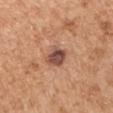Clinical impression:
This lesion was catalogued during total-body skin photography and was not selected for biopsy.
Acquisition and patient details:
Located on the left upper arm. A male subject about 65 years old. A 15 mm close-up extracted from a 3D total-body photography capture. The total-body-photography lesion software estimated a lesion area of about 6.5 mm², a shape eccentricity near 0.5, and a symmetry-axis asymmetry near 0.15. And it measured a lesion color around L≈50 a*≈22 b*≈28 in CIELAB, about 14 CIELAB-L* units darker than the surrounding skin, and a normalized lesion–skin contrast near 10. The tile uses white-light illumination. The recorded lesion diameter is about 3 mm.